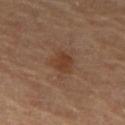Q: Is there a histopathology result?
A: catalogued during a skin exam; not biopsied
Q: How was this image acquired?
A: ~15 mm tile from a whole-body skin photo
Q: Lesion size?
A: about 3 mm
Q: Illumination type?
A: cross-polarized
Q: Lesion location?
A: the right thigh
Q: What did automated image analysis measure?
A: a lesion area of about 5.5 mm² and an eccentricity of roughly 0.45
Q: Who is the patient?
A: female, aged 58–62